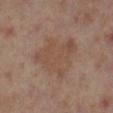Q: Was this lesion biopsied?
A: imaged on a skin check; not biopsied
Q: How was this image acquired?
A: ~15 mm tile from a whole-body skin photo
Q: Lesion size?
A: ~6 mm (longest diameter)
Q: What is the anatomic site?
A: the right lower leg
Q: Who is the patient?
A: female, about 40 years old
Q: Illumination type?
A: cross-polarized